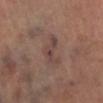| field | value |
|---|---|
| workup | catalogued during a skin exam; not biopsied |
| illumination | cross-polarized illumination |
| size | about 4 mm |
| automated metrics | an outline eccentricity of about 0.85 (0 = round, 1 = elongated) and a symmetry-axis asymmetry near 0.65; a lesion color around L≈43 a*≈18 b*≈21 in CIELAB, about 7 CIELAB-L* units darker than the surrounding skin, and a normalized border contrast of about 6; a color-variation rating of about 3.5/10 and radial color variation of about 1 |
| image source | 15 mm crop, total-body photography |
| anatomic site | the leg |